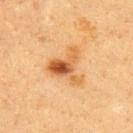Imaged during a routine full-body skin examination; the lesion was not biopsied and no histopathology is available. From the upper back. The tile uses cross-polarized illumination. Cropped from a total-body skin-imaging series; the visible field is about 15 mm. A female subject, roughly 40 years of age. Measured at roughly 4.5 mm in maximum diameter.